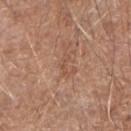Assessment: This lesion was catalogued during total-body skin photography and was not selected for biopsy. Background: An algorithmic analysis of the crop reported a mean CIELAB color near L≈52 a*≈21 b*≈31, about 6 CIELAB-L* units darker than the surrounding skin, and a lesion-to-skin contrast of about 5 (normalized; higher = more distinct). The analysis additionally found a border-irregularity rating of about 5.5/10, a color-variation rating of about 0/10, and a peripheral color-asymmetry measure near 0. It also reported lesion-presence confidence of about 85/100. A close-up tile cropped from a whole-body skin photograph, about 15 mm across. Imaged with white-light lighting. About 2.5 mm across. A male subject, in their mid-60s. On the right forearm.